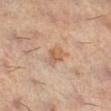follow-up: total-body-photography surveillance lesion; no biopsy | patient: female, in their 60s | tile lighting: cross-polarized illumination | anatomic site: the right lower leg | image source: ~15 mm tile from a whole-body skin photo | diameter: ~3.5 mm (longest diameter).